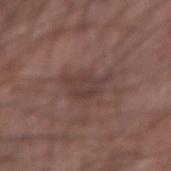This lesion was catalogued during total-body skin photography and was not selected for biopsy. A male subject, aged 63–67. A 15 mm close-up extracted from a 3D total-body photography capture. This is a white-light tile. Approximately 4.5 mm at its widest. From the left forearm.